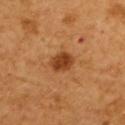Q: Was a biopsy performed?
A: catalogued during a skin exam; not biopsied
Q: What is the lesion's diameter?
A: ~3 mm (longest diameter)
Q: What is the imaging modality?
A: ~15 mm tile from a whole-body skin photo
Q: How was the tile lit?
A: cross-polarized
Q: Lesion location?
A: the back
Q: Who is the patient?
A: female, aged around 55